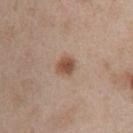Findings:
• biopsy status: total-body-photography surveillance lesion; no biopsy
• location: the front of the torso
• lesion size: ~2.5 mm (longest diameter)
• subject: female, aged around 40
• acquisition: 15 mm crop, total-body photography
• automated lesion analysis: a shape eccentricity near 0.6 and two-axis asymmetry of about 0.25; a lesion color around L≈51 a*≈19 b*≈28 in CIELAB, a lesion–skin lightness drop of about 12, and a lesion-to-skin contrast of about 8.5 (normalized; higher = more distinct)